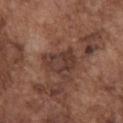biopsy status: catalogued during a skin exam; not biopsied | tile lighting: white-light illumination | subject: male, aged 73 to 77 | image-analysis metrics: an average lesion color of about L≈37 a*≈19 b*≈23 (CIELAB), a lesion–skin lightness drop of about 8, and a lesion-to-skin contrast of about 8 (normalized; higher = more distinct) | body site: the front of the torso | image source: ~15 mm crop, total-body skin-cancer survey | size: about 4 mm.